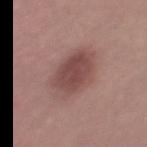workup = total-body-photography surveillance lesion; no biopsy
tile lighting = white-light illumination
location = the left thigh
image source = 15 mm crop, total-body photography
diameter = about 6.5 mm
subject = female, aged around 50
image-analysis metrics = border irregularity of about 2 on a 0–10 scale, a within-lesion color-variation index near 4.5/10, and peripheral color asymmetry of about 1.5; a nevus-likeness score of about 90/100 and lesion-presence confidence of about 100/100